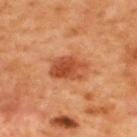<case>
  <biopsy_status>not biopsied; imaged during a skin examination</biopsy_status>
  <automated_metrics>
    <cielab_L>51</cielab_L>
    <cielab_a>31</cielab_a>
    <cielab_b>40</cielab_b>
    <vs_skin_darker_L>12.0</vs_skin_darker_L>
    <vs_skin_contrast_norm>8.5</vs_skin_contrast_norm>
  </automated_metrics>
  <site>upper back</site>
  <lighting>cross-polarized</lighting>
  <patient>
    <sex>male</sex>
    <age_approx>50</age_approx>
  </patient>
  <image>
    <source>total-body photography crop</source>
    <field_of_view_mm>15</field_of_view_mm>
  </image>
</case>